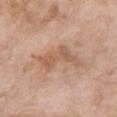From the chest. Imaged with white-light lighting. The patient is a female approximately 75 years of age. Cropped from a whole-body photographic skin survey; the tile spans about 15 mm.